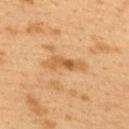automated lesion analysis: a lesion color around L≈48 a*≈18 b*≈36 in CIELAB and roughly 8 lightness units darker than nearby skin
anatomic site: the upper back
lesion size: ~4.5 mm (longest diameter)
tile lighting: cross-polarized
patient: female, approximately 40 years of age
acquisition: ~15 mm tile from a whole-body skin photo